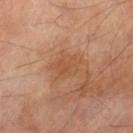{
  "biopsy_status": "not biopsied; imaged during a skin examination",
  "lesion_size": {
    "long_diameter_mm_approx": 4.0
  },
  "automated_metrics": {
    "area_mm2_approx": 7.5,
    "eccentricity": 0.7,
    "shape_asymmetry": 0.25
  },
  "patient": {
    "sex": "male",
    "age_approx": 70
  },
  "site": "left thigh",
  "lighting": "cross-polarized",
  "image": {
    "source": "total-body photography crop",
    "field_of_view_mm": 15
  }
}